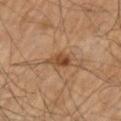Findings:
* workup: no biopsy performed (imaged during a skin exam)
* subject: male, aged around 60
* anatomic site: the right upper arm
* lighting: cross-polarized illumination
* diameter: ≈3 mm
* imaging modality: ~15 mm crop, total-body skin-cancer survey
* TBP lesion metrics: a lesion color around L≈41 a*≈18 b*≈31 in CIELAB, roughly 9 lightness units darker than nearby skin, and a normalized lesion–skin contrast near 8; border irregularity of about 2.5 on a 0–10 scale, internal color variation of about 4.5 on a 0–10 scale, and peripheral color asymmetry of about 2; a nevus-likeness score of about 80/100 and a lesion-detection confidence of about 100/100